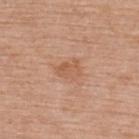Findings:
- workup: no biopsy performed (imaged during a skin exam)
- lesion size: ≈3 mm
- imaging modality: 15 mm crop, total-body photography
- location: the upper back
- automated lesion analysis: a lesion area of about 4.5 mm², an eccentricity of roughly 0.75, and a shape-asymmetry score of about 0.35 (0 = symmetric); a lesion color around L≈57 a*≈22 b*≈34 in CIELAB, about 7 CIELAB-L* units darker than the surrounding skin, and a lesion-to-skin contrast of about 5.5 (normalized; higher = more distinct); border irregularity of about 4 on a 0–10 scale, a color-variation rating of about 2/10, and peripheral color asymmetry of about 1; a lesion-detection confidence of about 100/100
- patient: female, aged around 65